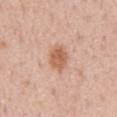follow-up: catalogued during a skin exam; not biopsied | patient: male, approximately 55 years of age | lighting: white-light illumination | acquisition: total-body-photography crop, ~15 mm field of view | lesion size: ≈3.5 mm | location: the abdomen | automated metrics: a footprint of about 7 mm², a shape eccentricity near 0.7, and a shape-asymmetry score of about 0.15 (0 = symmetric); an average lesion color of about L≈61 a*≈22 b*≈33 (CIELAB), roughly 11 lightness units darker than nearby skin, and a normalized border contrast of about 8; radial color variation of about 1; a lesion-detection confidence of about 100/100.